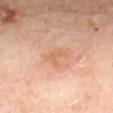follow-up: total-body-photography surveillance lesion; no biopsy
subject: female, roughly 60 years of age
imaging modality: total-body-photography crop, ~15 mm field of view
body site: the back